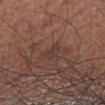Clinical impression:
The lesion was photographed on a routine skin check and not biopsied; there is no pathology result.
Background:
Automated tile analysis of the lesion measured a mean CIELAB color near L≈37 a*≈18 b*≈23, a lesion–skin lightness drop of about 5, and a normalized border contrast of about 5. And it measured a border-irregularity rating of about 3.5/10 and a within-lesion color-variation index near 1/10. The tile uses white-light illumination. Approximately 3 mm at its widest. The subject is a male aged around 65. The lesion is located on the arm. A 15 mm crop from a total-body photograph taken for skin-cancer surveillance.